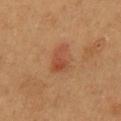Captured during whole-body skin photography for melanoma surveillance; the lesion was not biopsied. Cropped from a whole-body photographic skin survey; the tile spans about 15 mm. The lesion is located on the mid back. The lesion-visualizer software estimated about 7 CIELAB-L* units darker than the surrounding skin and a normalized border contrast of about 6. The analysis additionally found a classifier nevus-likeness of about 95/100. The patient is a female aged 38 to 42.